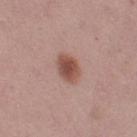Imaged during a routine full-body skin examination; the lesion was not biopsied and no histopathology is available. The subject is a female roughly 50 years of age. A 15 mm close-up tile from a total-body photography series done for melanoma screening. From the left thigh. This is a white-light tile. About 3.5 mm across.